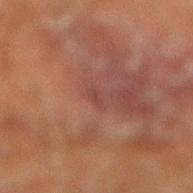Captured during whole-body skin photography for melanoma surveillance; the lesion was not biopsied.
This is a cross-polarized tile.
Located on the right lower leg.
An algorithmic analysis of the crop reported an area of roughly 1.5 mm². The software also gave a color-variation rating of about 0/10 and a peripheral color-asymmetry measure near 0. It also reported a lesion-detection confidence of about 90/100.
A close-up tile cropped from a whole-body skin photograph, about 15 mm across.
A male patient, aged 63 to 67.